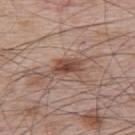Q: Was a biopsy performed?
A: no biopsy performed (imaged during a skin exam)
Q: Automated lesion metrics?
A: an average lesion color of about L≈48 a*≈20 b*≈25 (CIELAB); a border-irregularity index near 5/10 and radial color variation of about 1; a classifier nevus-likeness of about 90/100
Q: Lesion size?
A: ~4.5 mm (longest diameter)
Q: Lesion location?
A: the upper back
Q: How was this image acquired?
A: 15 mm crop, total-body photography
Q: What are the patient's age and sex?
A: male, aged 63 to 67
Q: How was the tile lit?
A: white-light illumination